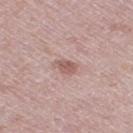Imaged during a routine full-body skin examination; the lesion was not biopsied and no histopathology is available. The subject is a female about 40 years old. A region of skin cropped from a whole-body photographic capture, roughly 15 mm wide. The tile uses white-light illumination. Longest diameter approximately 2.5 mm. The lesion is located on the right thigh.